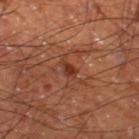Clinical impression:
This lesion was catalogued during total-body skin photography and was not selected for biopsy.
Context:
About 2 mm across. On the left thigh. The total-body-photography lesion software estimated an eccentricity of roughly 0.75 and a shape-asymmetry score of about 0.35 (0 = symmetric). It also reported a mean CIELAB color near L≈32 a*≈23 b*≈29 and roughly 8 lightness units darker than nearby skin. The analysis additionally found a border-irregularity index near 3/10, a color-variation rating of about 1/10, and radial color variation of about 0.5. The analysis additionally found a classifier nevus-likeness of about 20/100 and a detector confidence of about 100 out of 100 that the crop contains a lesion. A male patient about 60 years old. Captured under cross-polarized illumination. This image is a 15 mm lesion crop taken from a total-body photograph.